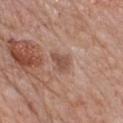The lesion was photographed on a routine skin check and not biopsied; there is no pathology result. The subject is a female aged 68–72. The recorded lesion diameter is about 2.5 mm. The tile uses white-light illumination. The total-body-photography lesion software estimated an area of roughly 3.5 mm², an eccentricity of roughly 0.8, and two-axis asymmetry of about 0.3. It also reported a border-irregularity rating of about 3/10. The analysis additionally found a classifier nevus-likeness of about 0/100 and a detector confidence of about 100 out of 100 that the crop contains a lesion. A close-up tile cropped from a whole-body skin photograph, about 15 mm across. The lesion is located on the chest.